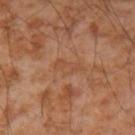notes: total-body-photography surveillance lesion; no biopsy | subject: male, approximately 55 years of age | lesion diameter: ~3.5 mm (longest diameter) | acquisition: ~15 mm crop, total-body skin-cancer survey | illumination: cross-polarized | location: the right forearm | automated metrics: an area of roughly 4.5 mm², an outline eccentricity of about 0.9 (0 = round, 1 = elongated), and a shape-asymmetry score of about 0.45 (0 = symmetric); a lesion color around L≈49 a*≈22 b*≈34 in CIELAB, roughly 5 lightness units darker than nearby skin, and a normalized lesion–skin contrast near 4.5.